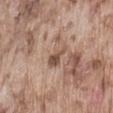follow-up = imaged on a skin check; not biopsied | location = the lower back | subject = male, about 75 years old | image = ~15 mm crop, total-body skin-cancer survey.